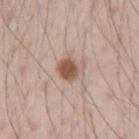notes = total-body-photography surveillance lesion; no biopsy
lighting = white-light
subject = male, aged 68 to 72
size = ~3 mm (longest diameter)
image source = 15 mm crop, total-body photography
site = the left upper arm